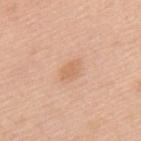Clinical impression: This lesion was catalogued during total-body skin photography and was not selected for biopsy. Acquisition and patient details: Automated tile analysis of the lesion measured a shape eccentricity near 0.8 and a shape-asymmetry score of about 0.2 (0 = symmetric). And it measured a lesion color around L≈65 a*≈23 b*≈34 in CIELAB and a normalized border contrast of about 5. It also reported an automated nevus-likeness rating near 60 out of 100. The subject is a female about 65 years old. The lesion is located on the back. Captured under white-light illumination. This image is a 15 mm lesion crop taken from a total-body photograph. Approximately 2.5 mm at its widest.